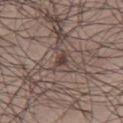The lesion was photographed on a routine skin check and not biopsied; there is no pathology result.
A male patient, in their mid- to late 60s.
A roughly 15 mm field-of-view crop from a total-body skin photograph.
The tile uses white-light illumination.
The lesion is located on the leg.